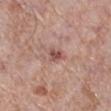  image:
    source: total-body photography crop
    field_of_view_mm: 15
  patient:
    sex: female
    age_approx: 70
  site: right lower leg
  lighting: white-light
  lesion_size:
    long_diameter_mm_approx: 2.5